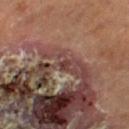biopsy status: total-body-photography surveillance lesion; no biopsy
subject: male, in their 70s
imaging modality: total-body-photography crop, ~15 mm field of view
location: the leg
TBP lesion metrics: a footprint of about 1.5 mm², an outline eccentricity of about 0.55 (0 = round, 1 = elongated), and a symmetry-axis asymmetry near 0.35; border irregularity of about 2.5 on a 0–10 scale and radial color variation of about 0